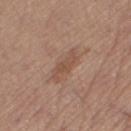notes=catalogued during a skin exam; not biopsied | anatomic site=the left thigh | automated lesion analysis=a lesion color around L≈51 a*≈19 b*≈28 in CIELAB and a lesion–skin lightness drop of about 7; a classifier nevus-likeness of about 0/100 and lesion-presence confidence of about 100/100 | lighting=white-light illumination | subject=female, approximately 65 years of age | imaging modality=~15 mm tile from a whole-body skin photo.